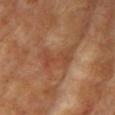Assessment:
Captured during whole-body skin photography for melanoma surveillance; the lesion was not biopsied.
Acquisition and patient details:
A close-up tile cropped from a whole-body skin photograph, about 15 mm across. A female subject, about 75 years old. Longest diameter approximately 4 mm. From the left upper arm. Captured under cross-polarized illumination.